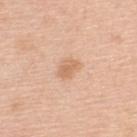Q: Was this lesion biopsied?
A: no biopsy performed (imaged during a skin exam)
Q: Patient demographics?
A: male, aged 48–52
Q: Where on the body is the lesion?
A: the upper back
Q: How large is the lesion?
A: ~3 mm (longest diameter)
Q: Illumination type?
A: white-light
Q: How was this image acquired?
A: 15 mm crop, total-body photography